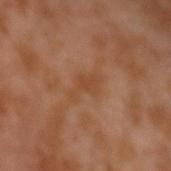Assessment: The lesion was tiled from a total-body skin photograph and was not biopsied. Image and clinical context: Automated tile analysis of the lesion measured a shape eccentricity near 0.85 and a shape-asymmetry score of about 0.45 (0 = symmetric). The software also gave a lesion color around L≈44 a*≈21 b*≈33 in CIELAB and a normalized lesion–skin contrast near 5. And it measured a classifier nevus-likeness of about 0/100 and a detector confidence of about 100 out of 100 that the crop contains a lesion. On the left upper arm. A 15 mm close-up tile from a total-body photography series done for melanoma screening. The patient is a male in their 30s. This is a cross-polarized tile. Approximately 3.5 mm at its widest.